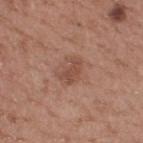anatomic site: the upper back | TBP lesion metrics: an area of roughly 4.5 mm²; a lesion color around L≈48 a*≈22 b*≈28 in CIELAB, a lesion–skin lightness drop of about 8, and a normalized border contrast of about 6; a border-irregularity rating of about 3.5/10, a color-variation rating of about 1/10, and a peripheral color-asymmetry measure near 0.5; a classifier nevus-likeness of about 10/100 and a lesion-detection confidence of about 100/100 | acquisition: 15 mm crop, total-body photography | subject: male, aged approximately 65.